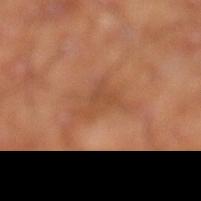Clinical impression:
Part of a total-body skin-imaging series; this lesion was reviewed on a skin check and was not flagged for biopsy.
Context:
The subject is a male aged around 60. The lesion-visualizer software estimated a mean CIELAB color near L≈47 a*≈23 b*≈34, about 7 CIELAB-L* units darker than the surrounding skin, and a normalized lesion–skin contrast near 5. The software also gave a border-irregularity index near 6/10, a within-lesion color-variation index near 1.5/10, and peripheral color asymmetry of about 0.5. A roughly 15 mm field-of-view crop from a total-body skin photograph. Measured at roughly 4 mm in maximum diameter. The tile uses cross-polarized illumination. From the leg.